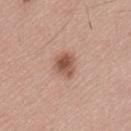biopsy_status: not biopsied; imaged during a skin examination
image:
  source: total-body photography crop
  field_of_view_mm: 15
lesion_size:
  long_diameter_mm_approx: 3.5
lighting: white-light
patient:
  sex: male
  age_approx: 55
site: back